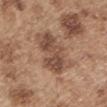biopsy_status: not biopsied; imaged during a skin examination
image:
  source: total-body photography crop
  field_of_view_mm: 15
site: left upper arm
lesion_size:
  long_diameter_mm_approx: 6.0
patient:
  sex: male
  age_approx: 55